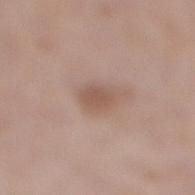| key | value |
|---|---|
| acquisition | ~15 mm tile from a whole-body skin photo |
| subject | male, about 70 years old |
| location | the right lower leg |